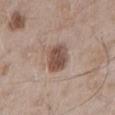Q: Is there a histopathology result?
A: no biopsy performed (imaged during a skin exam)
Q: What kind of image is this?
A: total-body-photography crop, ~15 mm field of view
Q: Where on the body is the lesion?
A: the right upper arm
Q: What are the patient's age and sex?
A: male, roughly 55 years of age